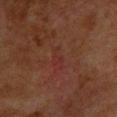Impression: The lesion was photographed on a routine skin check and not biopsied; there is no pathology result. Image and clinical context: The tile uses cross-polarized illumination. The lesion is located on the upper back. Cropped from a total-body skin-imaging series; the visible field is about 15 mm. The patient is a female roughly 80 years of age. Measured at roughly 3 mm in maximum diameter.